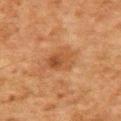Clinical impression:
This lesion was catalogued during total-body skin photography and was not selected for biopsy.
Acquisition and patient details:
A roughly 15 mm field-of-view crop from a total-body skin photograph. The lesion is located on the upper back. A male patient, roughly 80 years of age.